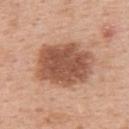  patient:
    sex: female
    age_approx: 50
  image:
    source: total-body photography crop
    field_of_view_mm: 15
  lesion_size:
    long_diameter_mm_approx: 7.0
  site: back